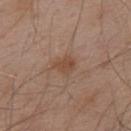Recorded during total-body skin imaging; not selected for excision or biopsy. On the upper back. Imaged with white-light lighting. An algorithmic analysis of the crop reported an area of roughly 4.5 mm² and a shape eccentricity near 0.6. It also reported a lesion color around L≈47 a*≈19 b*≈29 in CIELAB, a lesion–skin lightness drop of about 7, and a normalized border contrast of about 6.5. It also reported a border-irregularity rating of about 2/10 and radial color variation of about 0.5. And it measured an automated nevus-likeness rating near 10 out of 100 and a lesion-detection confidence of about 100/100. Approximately 2.5 mm at its widest. A male subject, aged 53 to 57. This image is a 15 mm lesion crop taken from a total-body photograph.